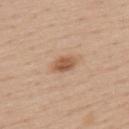The lesion was photographed on a routine skin check and not biopsied; there is no pathology result. Cropped from a total-body skin-imaging series; the visible field is about 15 mm. A male patient, in their mid- to late 50s. From the back.